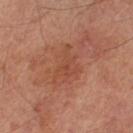– workup · imaged on a skin check; not biopsied
– patient · male, in their mid-60s
– image-analysis metrics · a shape eccentricity near 0.8 and two-axis asymmetry of about 0.5; a mean CIELAB color near L≈45 a*≈24 b*≈31, roughly 6 lightness units darker than nearby skin, and a normalized lesion–skin contrast near 5
– site · the left lower leg
– image source · 15 mm crop, total-body photography
– tile lighting · cross-polarized